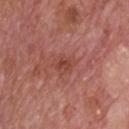biopsy status — catalogued during a skin exam; not biopsied | image source — ~15 mm crop, total-body skin-cancer survey | patient — male, aged 73 to 77 | illumination — white-light | body site — the upper back | lesion size — about 3.5 mm.